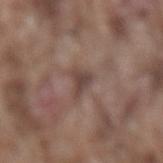<record>
  <biopsy_status>not biopsied; imaged during a skin examination</biopsy_status>
  <image>
    <source>total-body photography crop</source>
    <field_of_view_mm>15</field_of_view_mm>
  </image>
  <patient>
    <sex>male</sex>
    <age_approx>75</age_approx>
  </patient>
  <automated_metrics>
    <area_mm2_approx>3.5</area_mm2_approx>
    <eccentricity>0.7</eccentricity>
    <shape_asymmetry>0.5</shape_asymmetry>
    <cielab_L>43</cielab_L>
    <cielab_a>16</cielab_a>
    <cielab_b>21</cielab_b>
    <vs_skin_darker_L>9.0</vs_skin_darker_L>
    <vs_skin_contrast_norm>7.5</vs_skin_contrast_norm>
    <nevus_likeness_0_100>0</nevus_likeness_0_100>
    <lesion_detection_confidence_0_100>85</lesion_detection_confidence_0_100>
  </automated_metrics>
  <lighting>white-light</lighting>
  <site>back</site>
  <lesion_size>
    <long_diameter_mm_approx>3.0</long_diameter_mm_approx>
  </lesion_size>
</record>